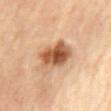{
  "biopsy_status": "not biopsied; imaged during a skin examination",
  "patient": {
    "sex": "female",
    "age_approx": 60
  },
  "image": {
    "source": "total-body photography crop",
    "field_of_view_mm": 15
  },
  "lesion_size": {
    "long_diameter_mm_approx": 4.5
  },
  "lighting": "cross-polarized",
  "site": "chest"
}